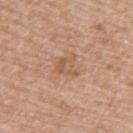follow-up: no biopsy performed (imaged during a skin exam)
subject: male, about 70 years old
image: total-body-photography crop, ~15 mm field of view
automated metrics: a lesion area of about 5.5 mm², an outline eccentricity of about 0.6 (0 = round, 1 = elongated), and a symmetry-axis asymmetry near 0.4; a border-irregularity index near 4.5/10, a color-variation rating of about 4/10, and peripheral color asymmetry of about 1
illumination: white-light illumination
site: the upper back